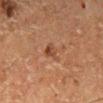Approximately 3 mm at its widest.
A 15 mm close-up extracted from a 3D total-body photography capture.
A female patient aged 48 to 52.
The lesion is on the left lower leg.